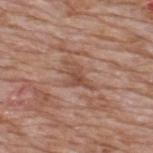<lesion>
<automated_metrics>
  <cielab_L>50</cielab_L>
  <cielab_a>21</cielab_a>
  <cielab_b>28</cielab_b>
  <vs_skin_darker_L>8.0</vs_skin_darker_L>
  <vs_skin_contrast_norm>6.0</vs_skin_contrast_norm>
</automated_metrics>
<image>
  <source>total-body photography crop</source>
  <field_of_view_mm>15</field_of_view_mm>
</image>
<patient>
  <sex>male</sex>
  <age_approx>60</age_approx>
</patient>
<site>upper back</site>
</lesion>